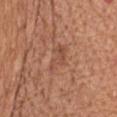Impression: Part of a total-body skin-imaging series; this lesion was reviewed on a skin check and was not flagged for biopsy. Background: Located on the chest. About 4.5 mm across. Cropped from a total-body skin-imaging series; the visible field is about 15 mm. The tile uses white-light illumination. Automated image analysis of the tile measured an outline eccentricity of about 0.9 (0 = round, 1 = elongated) and a symmetry-axis asymmetry near 0.4. It also reported an automated nevus-likeness rating near 0 out of 100. The patient is a male approximately 65 years of age.